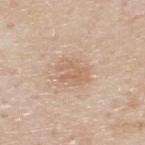Context:
Located on the back. A male subject about 80 years old. Longest diameter approximately 3.5 mm. A close-up tile cropped from a whole-body skin photograph, about 15 mm across.